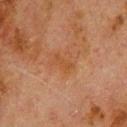Impression: This lesion was catalogued during total-body skin photography and was not selected for biopsy. Background: This image is a 15 mm lesion crop taken from a total-body photograph. A male patient, aged 78 to 82. The lesion is on the upper back.